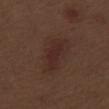Q: Was this lesion biopsied?
A: no biopsy performed (imaged during a skin exam)
Q: Who is the patient?
A: male, aged approximately 70
Q: Lesion size?
A: ≈4.5 mm
Q: What lighting was used for the tile?
A: white-light
Q: How was this image acquired?
A: ~15 mm tile from a whole-body skin photo
Q: Lesion location?
A: the right thigh
Q: What did automated image analysis measure?
A: border irregularity of about 4 on a 0–10 scale and a peripheral color-asymmetry measure near 0.5; a detector confidence of about 100 out of 100 that the crop contains a lesion